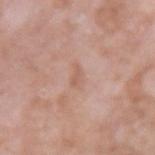No biopsy was performed on this lesion — it was imaged during a full skin examination and was not determined to be concerning.
A 15 mm crop from a total-body photograph taken for skin-cancer surveillance.
The subject is a male in their mid- to late 70s.
From the left upper arm.